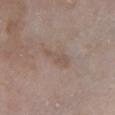Assessment:
This lesion was catalogued during total-body skin photography and was not selected for biopsy.
Background:
The tile uses white-light illumination. A region of skin cropped from a whole-body photographic capture, roughly 15 mm wide. The recorded lesion diameter is about 4 mm. Located on the leg. A female subject approximately 85 years of age or older.